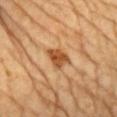Q: Was this lesion biopsied?
A: total-body-photography surveillance lesion; no biopsy